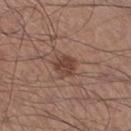Clinical impression: This lesion was catalogued during total-body skin photography and was not selected for biopsy. Acquisition and patient details: The lesion is located on the left lower leg. The recorded lesion diameter is about 2.5 mm. Cropped from a total-body skin-imaging series; the visible field is about 15 mm. Imaged with white-light lighting. A male subject roughly 55 years of age.